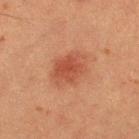Notes:
* workup · catalogued during a skin exam; not biopsied
* location · the right thigh
* image · total-body-photography crop, ~15 mm field of view
* tile lighting · cross-polarized illumination
* subject · female, aged 48–52